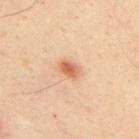* biopsy status — imaged on a skin check; not biopsied
* illumination — cross-polarized
* body site — the upper back
* automated metrics — a border-irregularity index near 2.5/10, internal color variation of about 3 on a 0–10 scale, and radial color variation of about 1; a nevus-likeness score of about 95/100 and lesion-presence confidence of about 100/100
* subject — male, roughly 50 years of age
* diameter — about 2.5 mm
* acquisition — total-body-photography crop, ~15 mm field of view